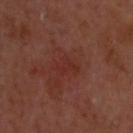notes: imaged on a skin check; not biopsied | image source: total-body-photography crop, ~15 mm field of view | subject: male, aged 58 to 62 | site: the head or neck.